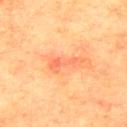<case>
<biopsy_status>not biopsied; imaged during a skin examination</biopsy_status>
<image>
  <source>total-body photography crop</source>
  <field_of_view_mm>15</field_of_view_mm>
</image>
<lesion_size>
  <long_diameter_mm_approx>4.5</long_diameter_mm_approx>
</lesion_size>
<patient>
  <sex>male</sex>
  <age_approx>75</age_approx>
</patient>
<site>upper back</site>
<automated_metrics>
  <area_mm2_approx>5.0</area_mm2_approx>
  <eccentricity>0.95</eccentricity>
  <shape_asymmetry>0.6</shape_asymmetry>
</automated_metrics>
<lighting>cross-polarized</lighting>
</case>